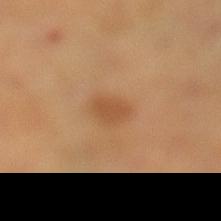follow-up — imaged on a skin check; not biopsied
illumination — cross-polarized
acquisition — total-body-photography crop, ~15 mm field of view
anatomic site — the leg
automated lesion analysis — an area of roughly 4.5 mm², a shape eccentricity near 0.75, and a symmetry-axis asymmetry near 0.25; a lesion-to-skin contrast of about 6 (normalized; higher = more distinct); a border-irregularity rating of about 2/10, internal color variation of about 1 on a 0–10 scale, and radial color variation of about 0.5
lesion diameter — about 3 mm
patient — male, roughly 55 years of age